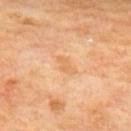biopsy status: imaged on a skin check; not biopsied
lesion diameter: ≈2.5 mm
image source: 15 mm crop, total-body photography
site: the back
patient: male, aged 68 to 72
automated metrics: a lesion color around L≈67 a*≈22 b*≈42 in CIELAB, a lesion–skin lightness drop of about 7, and a normalized lesion–skin contrast near 5; border irregularity of about 3 on a 0–10 scale and a within-lesion color-variation index near 0.5/10; a classifier nevus-likeness of about 0/100 and a detector confidence of about 100 out of 100 that the crop contains a lesion